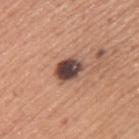Captured during whole-body skin photography for melanoma surveillance; the lesion was not biopsied. The lesion is on the left upper arm. Cropped from a total-body skin-imaging series; the visible field is about 15 mm. A male patient, in their mid-40s.